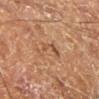Acquisition and patient details:
A 15 mm close-up tile from a total-body photography series done for melanoma screening. Captured under cross-polarized illumination. About 4 mm across. The lesion is located on the right leg. The patient is a male aged 58–62.